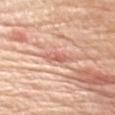{"biopsy_status": "not biopsied; imaged during a skin examination", "automated_metrics": {"cielab_L": 63, "cielab_a": 28, "cielab_b": 30, "vs_skin_darker_L": 10.0, "vs_skin_contrast_norm": 6.5, "nevus_likeness_0_100": 0, "lesion_detection_confidence_0_100": 75}, "image": {"source": "total-body photography crop", "field_of_view_mm": 15}, "patient": {"sex": "female", "age_approx": 70}, "lesion_size": {"long_diameter_mm_approx": 3.0}, "lighting": "white-light", "site": "left upper arm"}